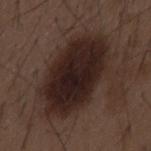Notes:
* biopsy status: catalogued during a skin exam; not biopsied
* subject: male, approximately 50 years of age
* body site: the mid back
* size: ~9.5 mm (longest diameter)
* image source: 15 mm crop, total-body photography